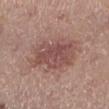This lesion was catalogued during total-body skin photography and was not selected for biopsy. Approximately 6.5 mm at its widest. Located on the leg. A male subject aged 53 to 57. The total-body-photography lesion software estimated a border-irregularity index near 3.5/10, internal color variation of about 4 on a 0–10 scale, and a peripheral color-asymmetry measure near 1.5. And it measured a classifier nevus-likeness of about 30/100. A roughly 15 mm field-of-view crop from a total-body skin photograph.